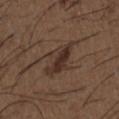Q: Where on the body is the lesion?
A: the chest
Q: How large is the lesion?
A: ≈4.5 mm
Q: How was this image acquired?
A: ~15 mm crop, total-body skin-cancer survey
Q: What are the patient's age and sex?
A: male, aged approximately 50
Q: What did automated image analysis measure?
A: a lesion area of about 9.5 mm² and an eccentricity of roughly 0.8; an average lesion color of about L≈32 a*≈15 b*≈22 (CIELAB), roughly 9 lightness units darker than nearby skin, and a lesion-to-skin contrast of about 8.5 (normalized; higher = more distinct); border irregularity of about 5 on a 0–10 scale, internal color variation of about 4.5 on a 0–10 scale, and radial color variation of about 1.5; a classifier nevus-likeness of about 45/100 and a lesion-detection confidence of about 100/100